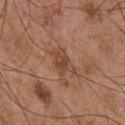Q: Was a biopsy performed?
A: total-body-photography surveillance lesion; no biopsy
Q: What is the anatomic site?
A: the chest
Q: What is the lesion's diameter?
A: ≈4 mm
Q: How was this image acquired?
A: ~15 mm crop, total-body skin-cancer survey
Q: Patient demographics?
A: male, aged around 55